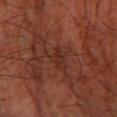biopsy_status: not biopsied; imaged during a skin examination
lighting: cross-polarized
image:
  source: total-body photography crop
  field_of_view_mm: 15
site: left thigh
lesion_size:
  long_diameter_mm_approx: 3.0
patient:
  age_approx: 65